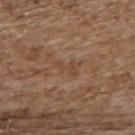A female patient in their mid- to late 60s. This is a white-light tile. A 15 mm close-up tile from a total-body photography series done for melanoma screening. Automated tile analysis of the lesion measured a lesion area of about 3.5 mm², an outline eccentricity of about 0.85 (0 = round, 1 = elongated), and a symmetry-axis asymmetry near 0.45. And it measured a lesion color around L≈45 a*≈17 b*≈29 in CIELAB, roughly 6 lightness units darker than nearby skin, and a normalized border contrast of about 4.5. The analysis additionally found an automated nevus-likeness rating near 0 out of 100 and lesion-presence confidence of about 55/100. The lesion is on the upper back. Approximately 3 mm at its widest.